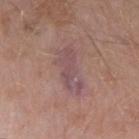{
  "biopsy_status": "not biopsied; imaged during a skin examination",
  "patient": {
    "sex": "male",
    "age_approx": "85+"
  },
  "lighting": "white-light",
  "lesion_size": {
    "long_diameter_mm_approx": 5.5
  },
  "image": {
    "source": "total-body photography crop",
    "field_of_view_mm": 15
  },
  "site": "left forearm"
}